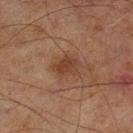Q: What is the anatomic site?
A: the left lower leg
Q: Patient demographics?
A: male, about 70 years old
Q: Automated lesion metrics?
A: an area of roughly 6 mm², an outline eccentricity of about 0.6 (0 = round, 1 = elongated), and a shape-asymmetry score of about 0.25 (0 = symmetric); a normalized lesion–skin contrast near 7
Q: How large is the lesion?
A: ≈3 mm
Q: Illumination type?
A: cross-polarized illumination
Q: What is the imaging modality?
A: total-body-photography crop, ~15 mm field of view